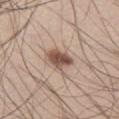notes = imaged on a skin check; not biopsied.